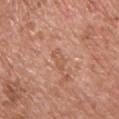Notes:
- notes: imaged on a skin check; not biopsied
- subject: male, aged approximately 70
- tile lighting: white-light illumination
- location: the chest
- lesion diameter: about 3 mm
- image-analysis metrics: a shape eccentricity near 0.9 and a symmetry-axis asymmetry near 0.25; roughly 6 lightness units darker than nearby skin and a normalized lesion–skin contrast near 5; a border-irregularity index near 3/10, internal color variation of about 1 on a 0–10 scale, and radial color variation of about 0.5; lesion-presence confidence of about 100/100
- acquisition: ~15 mm crop, total-body skin-cancer survey